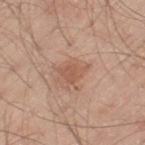Clinical impression: Recorded during total-body skin imaging; not selected for excision or biopsy. Context: Longest diameter approximately 3.5 mm. From the left thigh. Cropped from a whole-body photographic skin survey; the tile spans about 15 mm. An algorithmic analysis of the crop reported a footprint of about 5 mm², a shape eccentricity near 0.75, and a symmetry-axis asymmetry near 0.4. The subject is a male aged 58–62.